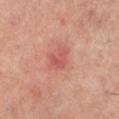Q: Was this lesion biopsied?
A: imaged on a skin check; not biopsied
Q: What are the patient's age and sex?
A: female, aged approximately 60
Q: Automated lesion metrics?
A: a lesion color around L≈57 a*≈32 b*≈28 in CIELAB, a lesion–skin lightness drop of about 9, and a normalized lesion–skin contrast near 5.5; border irregularity of about 4 on a 0–10 scale, internal color variation of about 2.5 on a 0–10 scale, and a peripheral color-asymmetry measure near 0.5
Q: Where on the body is the lesion?
A: the right lower leg
Q: How large is the lesion?
A: ~2.5 mm (longest diameter)
Q: Illumination type?
A: cross-polarized
Q: What kind of image is this?
A: 15 mm crop, total-body photography